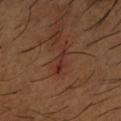Part of a total-body skin-imaging series; this lesion was reviewed on a skin check and was not flagged for biopsy. This is a cross-polarized tile. A male subject, approximately 65 years of age. A 15 mm close-up extracted from a 3D total-body photography capture. Located on the right forearm. An algorithmic analysis of the crop reported an average lesion color of about L≈31 a*≈23 b*≈26 (CIELAB). And it measured a border-irregularity rating of about 5.5/10, internal color variation of about 1 on a 0–10 scale, and radial color variation of about 0. Measured at roughly 3.5 mm in maximum diameter.